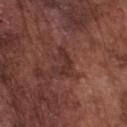The lesion was tiled from a total-body skin photograph and was not biopsied. Located on the chest. Longest diameter approximately 3.5 mm. The total-body-photography lesion software estimated an average lesion color of about L≈32 a*≈22 b*≈21 (CIELAB) and a normalized border contrast of about 6.5. The analysis additionally found a border-irregularity index near 7.5/10, internal color variation of about 0.5 on a 0–10 scale, and radial color variation of about 0. A region of skin cropped from a whole-body photographic capture, roughly 15 mm wide. The patient is a male in their mid- to late 70s.